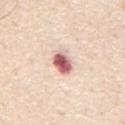Q: Is there a histopathology result?
A: no biopsy performed (imaged during a skin exam)